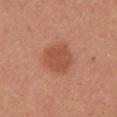Part of a total-body skin-imaging series; this lesion was reviewed on a skin check and was not flagged for biopsy.
The total-body-photography lesion software estimated a nevus-likeness score of about 85/100 and a detector confidence of about 100 out of 100 that the crop contains a lesion.
The patient is a female in their mid-20s.
This image is a 15 mm lesion crop taken from a total-body photograph.
On the left upper arm.
The lesion's longest dimension is about 3.5 mm.
Captured under white-light illumination.